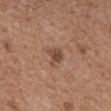{
  "biopsy_status": "not biopsied; imaged during a skin examination",
  "image": {
    "source": "total-body photography crop",
    "field_of_view_mm": 15
  },
  "patient": {
    "sex": "male",
    "age_approx": 65
  },
  "site": "mid back"
}